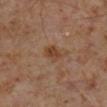The lesion was photographed on a routine skin check and not biopsied; there is no pathology result.
From the right lower leg.
Cropped from a total-body skin-imaging series; the visible field is about 15 mm.
The patient is a male about 60 years old.
Automated tile analysis of the lesion measured an average lesion color of about L≈38 a*≈18 b*≈29 (CIELAB), about 8 CIELAB-L* units darker than the surrounding skin, and a lesion-to-skin contrast of about 7.5 (normalized; higher = more distinct). It also reported a border-irregularity rating of about 1.5/10 and peripheral color asymmetry of about 1. The software also gave lesion-presence confidence of about 100/100.